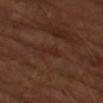Recorded during total-body skin imaging; not selected for excision or biopsy.